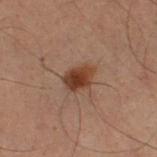Assessment:
No biopsy was performed on this lesion — it was imaged during a full skin examination and was not determined to be concerning.